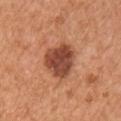Q: Was a biopsy performed?
A: imaged on a skin check; not biopsied
Q: Where on the body is the lesion?
A: the chest
Q: Automated lesion metrics?
A: an average lesion color of about L≈46 a*≈27 b*≈32 (CIELAB), a lesion–skin lightness drop of about 15, and a normalized border contrast of about 10.5; a border-irregularity rating of about 2.5/10, a within-lesion color-variation index near 4.5/10, and a peripheral color-asymmetry measure near 1.5
Q: How was this image acquired?
A: 15 mm crop, total-body photography
Q: Who is the patient?
A: female, aged approximately 45
Q: How large is the lesion?
A: ~4 mm (longest diameter)